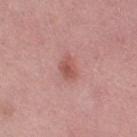  biopsy_status: not biopsied; imaged during a skin examination
  automated_metrics:
    cielab_L: 54
    cielab_a: 27
    cielab_b: 25
    vs_skin_darker_L: 9.0
    vs_skin_contrast_norm: 7.0
    peripheral_color_asymmetry: 0.5
    nevus_likeness_0_100: 45
    lesion_detection_confidence_0_100: 100
  image:
    source: total-body photography crop
    field_of_view_mm: 15
  patient:
    sex: female
    age_approx: 50
  lighting: white-light
  lesion_size:
    long_diameter_mm_approx: 3.0
  site: left thigh